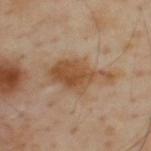Recorded during total-body skin imaging; not selected for excision or biopsy.
A male patient aged around 55.
A 15 mm crop from a total-body photograph taken for skin-cancer surveillance.
The lesion is located on the upper back.
Captured under cross-polarized illumination.
The lesion's longest dimension is about 7 mm.